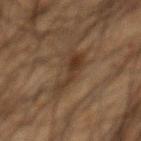{"biopsy_status": "not biopsied; imaged during a skin examination", "patient": {"sex": "male", "age_approx": 65}, "lighting": "cross-polarized", "image": {"source": "total-body photography crop", "field_of_view_mm": 15}, "site": "chest", "lesion_size": {"long_diameter_mm_approx": 5.0}}